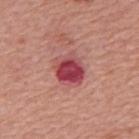notes=catalogued during a skin exam; not biopsied | acquisition=total-body-photography crop, ~15 mm field of view | site=the mid back | lesion size=about 4 mm | patient=male, in their mid-60s.